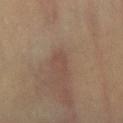biopsy status: total-body-photography surveillance lesion; no biopsy | image-analysis metrics: an automated nevus-likeness rating near 0 out of 100 and a lesion-detection confidence of about 95/100 | size: about 2.5 mm | location: the chest | subject: female, roughly 40 years of age | image source: total-body-photography crop, ~15 mm field of view.